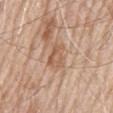Clinical impression:
The lesion was tiled from a total-body skin photograph and was not biopsied.
Background:
A male subject, aged 78 to 82. On the back. A lesion tile, about 15 mm wide, cut from a 3D total-body photograph. Longest diameter approximately 3 mm.